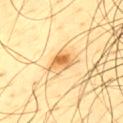Impression: The lesion was tiled from a total-body skin photograph and was not biopsied. Background: Measured at roughly 3.5 mm in maximum diameter. The subject is a male aged around 45. Cropped from a whole-body photographic skin survey; the tile spans about 15 mm. The tile uses cross-polarized illumination. Located on the upper back. Automated image analysis of the tile measured border irregularity of about 3.5 on a 0–10 scale, internal color variation of about 6 on a 0–10 scale, and a peripheral color-asymmetry measure near 1.5. It also reported an automated nevus-likeness rating near 85 out of 100 and lesion-presence confidence of about 100/100.